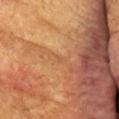* biopsy status — no biopsy performed (imaged during a skin exam)
* location — the head or neck
* image-analysis metrics — border irregularity of about 2.5 on a 0–10 scale, a within-lesion color-variation index near 0/10, and peripheral color asymmetry of about 0; a classifier nevus-likeness of about 0/100 and lesion-presence confidence of about 75/100
* patient — female, in their 70s
* illumination — cross-polarized illumination
* imaging modality — total-body-photography crop, ~15 mm field of view
* lesion size — about 1 mm